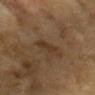No biopsy was performed on this lesion — it was imaged during a full skin examination and was not determined to be concerning. The tile uses cross-polarized illumination. Longest diameter approximately 3.5 mm. An algorithmic analysis of the crop reported a footprint of about 5 mm², an eccentricity of roughly 0.85, and a shape-asymmetry score of about 0.3 (0 = symmetric). The analysis additionally found a mean CIELAB color near L≈32 a*≈14 b*≈26, a lesion–skin lightness drop of about 6, and a lesion-to-skin contrast of about 6 (normalized; higher = more distinct). Cropped from a whole-body photographic skin survey; the tile spans about 15 mm. A female subject approximately 60 years of age. From the head or neck.